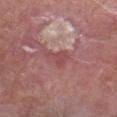biopsy_status: not biopsied; imaged during a skin examination
image:
  source: total-body photography crop
  field_of_view_mm: 15
automated_metrics:
  area_mm2_approx: 4.5
  eccentricity: 0.7
  shape_asymmetry: 0.5
  border_irregularity_0_10: 5.0
  color_variation_0_10: 1.0
site: leg
patient:
  sex: male
  age_approx: 65